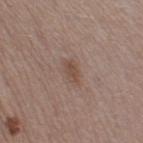Recorded during total-body skin imaging; not selected for excision or biopsy.
Cropped from a whole-body photographic skin survey; the tile spans about 15 mm.
Approximately 3 mm at its widest.
On the right thigh.
A female subject in their 40s.
The tile uses white-light illumination.